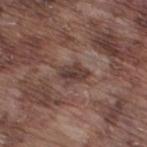The lesion was tiled from a total-body skin photograph and was not biopsied. A 15 mm crop from a total-body photograph taken for skin-cancer surveillance. Captured under white-light illumination. Located on the right thigh. The subject is a male aged 73–77.